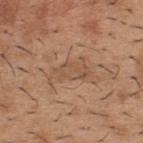No biopsy was performed on this lesion — it was imaged during a full skin examination and was not determined to be concerning. A 15 mm close-up extracted from a 3D total-body photography capture. From the back. A male subject aged around 40. The tile uses white-light illumination. About 4 mm across.